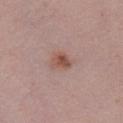Assessment:
The lesion was tiled from a total-body skin photograph and was not biopsied.
Background:
This is a white-light tile. The total-body-photography lesion software estimated an area of roughly 4 mm², a shape eccentricity near 0.55, and a shape-asymmetry score of about 0.3 (0 = symmetric). The software also gave an automated nevus-likeness rating near 85 out of 100. A female patient aged 43–47. A close-up tile cropped from a whole-body skin photograph, about 15 mm across. The lesion is on the chest.